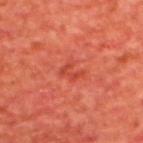Q: Is there a histopathology result?
A: imaged on a skin check; not biopsied
Q: Automated lesion metrics?
A: a lesion color around L≈44 a*≈37 b*≈34 in CIELAB and roughly 6 lightness units darker than nearby skin; a border-irregularity index near 5.5/10, a color-variation rating of about 1.5/10, and peripheral color asymmetry of about 0.5; a nevus-likeness score of about 0/100 and a detector confidence of about 100 out of 100 that the crop contains a lesion
Q: What is the anatomic site?
A: the back
Q: Patient demographics?
A: male, aged approximately 65
Q: How large is the lesion?
A: about 2.5 mm
Q: How was the tile lit?
A: cross-polarized
Q: What is the imaging modality?
A: 15 mm crop, total-body photography